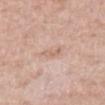Impression:
This lesion was catalogued during total-body skin photography and was not selected for biopsy.
Clinical summary:
A male subject aged 58–62. Imaged with white-light lighting. The lesion is on the abdomen. Longest diameter approximately 2.5 mm. A 15 mm close-up tile from a total-body photography series done for melanoma screening. Automated image analysis of the tile measured a footprint of about 2.5 mm² and an eccentricity of roughly 0.85. It also reported a lesion color around L≈64 a*≈19 b*≈28 in CIELAB, about 7 CIELAB-L* units darker than the surrounding skin, and a lesion-to-skin contrast of about 5 (normalized; higher = more distinct).